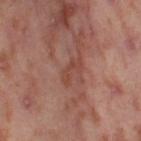This lesion was catalogued during total-body skin photography and was not selected for biopsy.
Cropped from a total-body skin-imaging series; the visible field is about 15 mm.
A female subject roughly 55 years of age.
The lesion is on the leg.